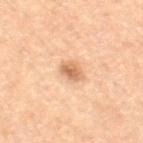{"biopsy_status": "not biopsied; imaged during a skin examination", "site": "right thigh", "image": {"source": "total-body photography crop", "field_of_view_mm": 15}, "lighting": "cross-polarized", "patient": {"sex": "female", "age_approx": 55}, "lesion_size": {"long_diameter_mm_approx": 2.5}}